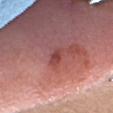Clinical summary: A lesion tile, about 15 mm wide, cut from a 3D total-body photograph. The subject is a female roughly 40 years of age. From the head or neck.